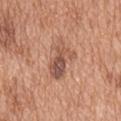{
  "biopsy_status": "not biopsied; imaged during a skin examination",
  "automated_metrics": {
    "area_mm2_approx": 10.0,
    "eccentricity": 0.85,
    "vs_skin_darker_L": 11.0,
    "vs_skin_contrast_norm": 8.0,
    "border_irregularity_0_10": 5.0,
    "color_variation_0_10": 8.0,
    "peripheral_color_asymmetry": 3.0
  },
  "patient": {
    "sex": "male",
    "age_approx": 65
  },
  "image": {
    "source": "total-body photography crop",
    "field_of_view_mm": 15
  },
  "site": "back",
  "lighting": "white-light"
}